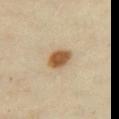| field | value |
|---|---|
| image source | total-body-photography crop, ~15 mm field of view |
| diameter | about 3 mm |
| lighting | cross-polarized |
| image-analysis metrics | an area of roughly 6 mm²; a border-irregularity index near 1/10, a within-lesion color-variation index near 3/10, and peripheral color asymmetry of about 1; an automated nevus-likeness rating near 100 out of 100 |
| location | the chest |
| subject | female, approximately 35 years of age |